This image is a 15 mm lesion crop taken from a total-body photograph, on the chest, the subject is a female roughly 45 years of age, this is a cross-polarized tile, Automated image analysis of the tile measured a nevus-likeness score of about 0/100 and a lesion-detection confidence of about 100/100, the lesion's longest dimension is about 1 mm:
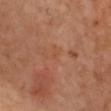On excision, pathology confirmed an actinic keratosis (borderline).